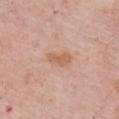Assessment:
No biopsy was performed on this lesion — it was imaged during a full skin examination and was not determined to be concerning.
Context:
Cropped from a whole-body photographic skin survey; the tile spans about 15 mm. About 3 mm across. The lesion is on the chest. This is a white-light tile. A female subject, aged approximately 65.